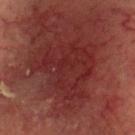biopsy status: no biopsy performed (imaged during a skin exam); image: 15 mm crop, total-body photography; lesion size: about 8 mm; patient: male, about 70 years old; lighting: cross-polarized; site: the head or neck.